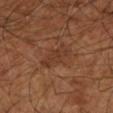follow-up = total-body-photography surveillance lesion; no biopsy
anatomic site = the left lower leg
lesion diameter = about 4.5 mm
image = total-body-photography crop, ~15 mm field of view
lighting = cross-polarized illumination
patient = approximately 65 years of age